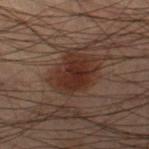Q: Was this lesion biopsied?
A: total-body-photography surveillance lesion; no biopsy